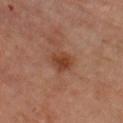{"biopsy_status": "not biopsied; imaged during a skin examination", "site": "left forearm", "patient": {"sex": "male", "age_approx": 70}, "image": {"source": "total-body photography crop", "field_of_view_mm": 15}, "lesion_size": {"long_diameter_mm_approx": 3.0}, "lighting": "cross-polarized"}